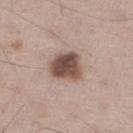<tbp_lesion>
  <biopsy_status>not biopsied; imaged during a skin examination</biopsy_status>
  <lesion_size>
    <long_diameter_mm_approx>4.0</long_diameter_mm_approx>
  </lesion_size>
  <image>
    <source>total-body photography crop</source>
    <field_of_view_mm>15</field_of_view_mm>
  </image>
  <lighting>white-light</lighting>
  <site>right lower leg</site>
  <patient>
    <sex>male</sex>
    <age_approx>60</age_approx>
  </patient>
  <automated_metrics>
    <cielab_L>50</cielab_L>
    <cielab_a>17</cielab_a>
    <cielab_b>23</cielab_b>
    <vs_skin_darker_L>16.0</vs_skin_darker_L>
    <vs_skin_contrast_norm>11.0</vs_skin_contrast_norm>
    <border_irregularity_0_10>2.0</border_irregularity_0_10>
    <color_variation_0_10>5.5</color_variation_0_10>
    <peripheral_color_asymmetry>1.5</peripheral_color_asymmetry>
  </automated_metrics>
</tbp_lesion>